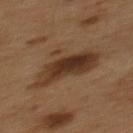Imaged during a routine full-body skin examination; the lesion was not biopsied and no histopathology is available. Longest diameter approximately 7.5 mm. The subject is a male in their mid- to late 50s. From the mid back. Captured under cross-polarized illumination. Cropped from a total-body skin-imaging series; the visible field is about 15 mm.